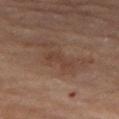Captured during whole-body skin photography for melanoma surveillance; the lesion was not biopsied.